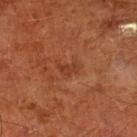A 15 mm close-up tile from a total-body photography series done for melanoma screening. The tile uses cross-polarized illumination. Located on the left lower leg. The lesion's longest dimension is about 3 mm. A male subject in their mid-60s.